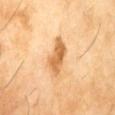Notes:
• biopsy status: total-body-photography surveillance lesion; no biopsy
• location: the right thigh
• imaging modality: ~15 mm crop, total-body skin-cancer survey
• illumination: cross-polarized illumination
• patient: male, about 60 years old